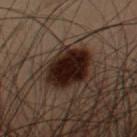Case summary:
* biopsy status · total-body-photography surveillance lesion; no biopsy
* body site · the left upper arm
* lesion size · ≈5.5 mm
* image source · ~15 mm tile from a whole-body skin photo
* patient · male, aged 53–57